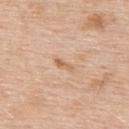  biopsy_status: not biopsied; imaged during a skin examination
  patient:
    sex: male
    age_approx: 60
  image:
    source: total-body photography crop
    field_of_view_mm: 15
  lesion_size:
    long_diameter_mm_approx: 2.5
  site: upper back
  lighting: white-light